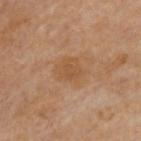The lesion is on the upper back. A lesion tile, about 15 mm wide, cut from a 3D total-body photograph. A male patient aged 63 to 67. Approximately 3.5 mm at its widest.